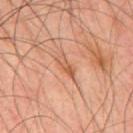Q: Is there a histopathology result?
A: catalogued during a skin exam; not biopsied
Q: Patient demographics?
A: male, in their mid- to late 40s
Q: What kind of image is this?
A: ~15 mm crop, total-body skin-cancer survey
Q: What is the anatomic site?
A: the mid back